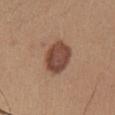{"biopsy_status": "not biopsied; imaged during a skin examination", "image": {"source": "total-body photography crop", "field_of_view_mm": 15}, "site": "right lower leg", "lighting": "white-light", "automated_metrics": {"eccentricity": 0.55}, "patient": {"sex": "male", "age_approx": 35}}